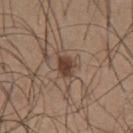<case>
<biopsy_status>not biopsied; imaged during a skin examination</biopsy_status>
<site>chest</site>
<patient>
  <sex>male</sex>
  <age_approx>25</age_approx>
</patient>
<image>
  <source>total-body photography crop</source>
  <field_of_view_mm>15</field_of_view_mm>
</image>
<lesion_size>
  <long_diameter_mm_approx>2.5</long_diameter_mm_approx>
</lesion_size>
<lighting>white-light</lighting>
</case>